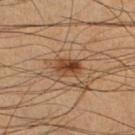notes = imaged on a skin check; not biopsied
image-analysis metrics = a footprint of about 6 mm², an eccentricity of roughly 0.7, and a symmetry-axis asymmetry near 0.2; a color-variation rating of about 7/10 and radial color variation of about 2.5
acquisition = ~15 mm crop, total-body skin-cancer survey
patient = male, approximately 40 years of age
lighting = cross-polarized illumination
site = the right lower leg